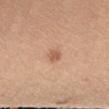Imaged during a routine full-body skin examination; the lesion was not biopsied and no histopathology is available.
The lesion is on the front of the torso.
Imaged with white-light lighting.
The recorded lesion diameter is about 3 mm.
Automated tile analysis of the lesion measured a lesion area of about 5.5 mm² and two-axis asymmetry of about 0.15. It also reported a border-irregularity rating of about 1.5/10, internal color variation of about 3.5 on a 0–10 scale, and a peripheral color-asymmetry measure near 1.
A female subject roughly 45 years of age.
This image is a 15 mm lesion crop taken from a total-body photograph.